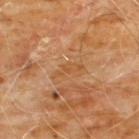biopsy_status: not biopsied; imaged during a skin examination
lighting: cross-polarized
site: front of the torso
automated_metrics:
  area_mm2_approx: 3.5
  eccentricity: 0.95
  shape_asymmetry: 0.4
image:
  source: total-body photography crop
  field_of_view_mm: 15
lesion_size:
  long_diameter_mm_approx: 3.5
patient:
  sex: male
  age_approx: 60